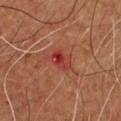The lesion was tiled from a total-body skin photograph and was not biopsied. Captured under cross-polarized illumination. A male patient, aged approximately 65. The lesion is located on the chest. An algorithmic analysis of the crop reported a footprint of about 3.5 mm² and a shape eccentricity near 0.8. The software also gave border irregularity of about 4 on a 0–10 scale, internal color variation of about 2.5 on a 0–10 scale, and a peripheral color-asymmetry measure near 0.5. It also reported an automated nevus-likeness rating near 0 out of 100 and a detector confidence of about 100 out of 100 that the crop contains a lesion. Cropped from a whole-body photographic skin survey; the tile spans about 15 mm. Longest diameter approximately 2.5 mm.